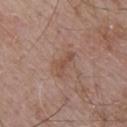The lesion was tiled from a total-body skin photograph and was not biopsied. A roughly 15 mm field-of-view crop from a total-body skin photograph. The recorded lesion diameter is about 3.5 mm. A male patient, aged approximately 55. An algorithmic analysis of the crop reported about 7 CIELAB-L* units darker than the surrounding skin and a normalized border contrast of about 5.5. The analysis additionally found a border-irregularity rating of about 5.5/10 and peripheral color asymmetry of about 0.5. It also reported a classifier nevus-likeness of about 0/100 and a detector confidence of about 100 out of 100 that the crop contains a lesion. The tile uses white-light illumination. The lesion is on the upper back.